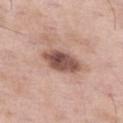follow-up: no biopsy performed (imaged during a skin exam) | patient: male, aged 53 to 57 | image: ~15 mm crop, total-body skin-cancer survey | body site: the left thigh.